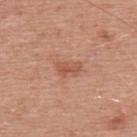{
  "biopsy_status": "not biopsied; imaged during a skin examination",
  "image": {
    "source": "total-body photography crop",
    "field_of_view_mm": 15
  },
  "patient": {
    "sex": "male",
    "age_approx": 50
  },
  "lighting": "white-light",
  "automated_metrics": {
    "vs_skin_darker_L": 8.0,
    "vs_skin_contrast_norm": 6.0,
    "border_irregularity_0_10": 4.0,
    "color_variation_0_10": 2.5,
    "peripheral_color_asymmetry": 1.0
  },
  "site": "upper back",
  "lesion_size": {
    "long_diameter_mm_approx": 3.0
  }
}